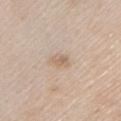Q: Is there a histopathology result?
A: catalogued during a skin exam; not biopsied